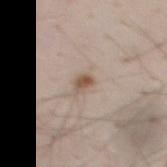Clinical impression: The lesion was photographed on a routine skin check and not biopsied; there is no pathology result. Background: Cropped from a whole-body photographic skin survey; the tile spans about 15 mm. Automated image analysis of the tile measured a shape eccentricity near 0.8 and two-axis asymmetry of about 0.35. On the mid back. A male patient, approximately 35 years of age.